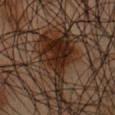Findings:
• workup — total-body-photography surveillance lesion; no biopsy
• tile lighting — cross-polarized
• automated metrics — a lesion area of about 22 mm², a shape eccentricity near 0.8, and a shape-asymmetry score of about 0.6 (0 = symmetric); a mean CIELAB color near L≈23 a*≈16 b*≈23, roughly 11 lightness units darker than nearby skin, and a lesion-to-skin contrast of about 12 (normalized; higher = more distinct); border irregularity of about 9 on a 0–10 scale, a color-variation rating of about 5.5/10, and a peripheral color-asymmetry measure near 2; an automated nevus-likeness rating near 100 out of 100
• size — about 9 mm
• anatomic site — the chest
• acquisition — total-body-photography crop, ~15 mm field of view
• subject — male, approximately 45 years of age